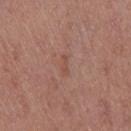The subject is a female aged 38–42. The recorded lesion diameter is about 2.5 mm. On the right thigh. A 15 mm close-up extracted from a 3D total-body photography capture. An algorithmic analysis of the crop reported a mean CIELAB color near L≈50 a*≈21 b*≈27 and a lesion–skin lightness drop of about 6. The analysis additionally found border irregularity of about 4.5 on a 0–10 scale. The tile uses white-light illumination.